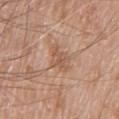- follow-up · no biopsy performed (imaged during a skin exam)
- subject · male, aged 78 to 82
- site · the chest
- image · total-body-photography crop, ~15 mm field of view